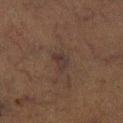Recorded during total-body skin imaging; not selected for excision or biopsy. A male patient aged 73–77. Longest diameter approximately 2.5 mm. This is a cross-polarized tile. The lesion is located on the left lower leg. A lesion tile, about 15 mm wide, cut from a 3D total-body photograph. Automated image analysis of the tile measured a footprint of about 3.5 mm². The software also gave a lesion color around L≈26 a*≈12 b*≈16 in CIELAB, about 5 CIELAB-L* units darker than the surrounding skin, and a lesion-to-skin contrast of about 7 (normalized; higher = more distinct).